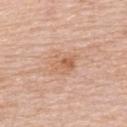Imaged during a routine full-body skin examination; the lesion was not biopsied and no histopathology is available. Captured under white-light illumination. A lesion tile, about 15 mm wide, cut from a 3D total-body photograph. Approximately 4 mm at its widest. The lesion is located on the upper back. A female patient, aged 48–52.